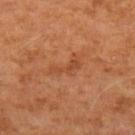  biopsy_status: not biopsied; imaged during a skin examination
  patient:
    sex: male
    age_approx: 55
  lighting: cross-polarized
  image:
    source: total-body photography crop
    field_of_view_mm: 15
  site: arm
  lesion_size:
    long_diameter_mm_approx: 3.5